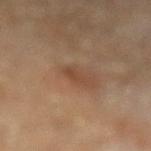{"biopsy_status": "not biopsied; imaged during a skin examination", "image": {"source": "total-body photography crop", "field_of_view_mm": 15}, "patient": {"age_approx": 55}, "site": "left lower leg"}